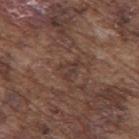The lesion was photographed on a routine skin check and not biopsied; there is no pathology result. The subject is a male roughly 75 years of age. Located on the mid back. A 15 mm close-up tile from a total-body photography series done for melanoma screening.